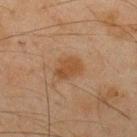The lesion was tiled from a total-body skin photograph and was not biopsied.